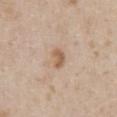{
  "biopsy_status": "not biopsied; imaged during a skin examination",
  "site": "chest",
  "image": {
    "source": "total-body photography crop",
    "field_of_view_mm": 15
  },
  "patient": {
    "sex": "female",
    "age_approx": 30
  },
  "automated_metrics": {
    "border_irregularity_0_10": 2.0,
    "color_variation_0_10": 2.0,
    "peripheral_color_asymmetry": 0.5
  }
}